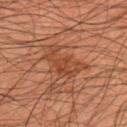Imaged during a routine full-body skin examination; the lesion was not biopsied and no histopathology is available.
A male subject aged 43 to 47.
Automated image analysis of the tile measured roughly 7 lightness units darker than nearby skin and a normalized border contrast of about 6. The analysis additionally found a color-variation rating of about 3/10 and a peripheral color-asymmetry measure near 1. The analysis additionally found a lesion-detection confidence of about 100/100.
Imaged with cross-polarized lighting.
The lesion's longest dimension is about 5.5 mm.
The lesion is on the upper back.
A 15 mm close-up tile from a total-body photography series done for melanoma screening.